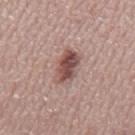Findings:
• biopsy status · total-body-photography surveillance lesion; no biopsy
• image source · ~15 mm crop, total-body skin-cancer survey
• subject · male, aged 68 to 72
• location · the mid back
• diameter · ~4.5 mm (longest diameter)
• lighting · white-light illumination
• TBP lesion metrics · a lesion area of about 9 mm², an eccentricity of roughly 0.85, and two-axis asymmetry of about 0.25; a lesion color around L≈50 a*≈21 b*≈21 in CIELAB and a lesion–skin lightness drop of about 13